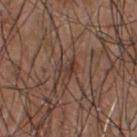body site: the chest; lighting: white-light illumination; imaging modality: ~15 mm crop, total-body skin-cancer survey; subject: male, roughly 55 years of age.